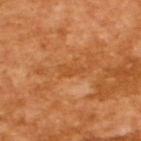The lesion was tiled from a total-body skin photograph and was not biopsied.